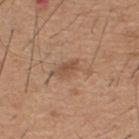{"biopsy_status": "not biopsied; imaged during a skin examination", "automated_metrics": {"area_mm2_approx": 7.5, "eccentricity": 0.9, "shape_asymmetry": 0.45, "vs_skin_darker_L": 8.0, "vs_skin_contrast_norm": 5.5, "border_irregularity_0_10": 6.0, "color_variation_0_10": 3.0, "peripheral_color_asymmetry": 1.0, "nevus_likeness_0_100": 5}, "patient": {"sex": "male", "age_approx": 60}, "lesion_size": {"long_diameter_mm_approx": 5.0}, "lighting": "white-light", "image": {"source": "total-body photography crop", "field_of_view_mm": 15}, "site": "upper back"}